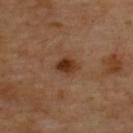The lesion was photographed on a routine skin check and not biopsied; there is no pathology result. This is a cross-polarized tile. A 15 mm close-up tile from a total-body photography series done for melanoma screening. On the back. The patient is aged around 65. The recorded lesion diameter is about 2.5 mm.